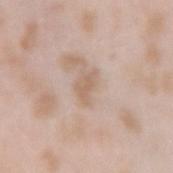Background:
Automated image analysis of the tile measured a mean CIELAB color near L≈62 a*≈16 b*≈28, roughly 8 lightness units darker than nearby skin, and a normalized lesion–skin contrast near 6. The software also gave a border-irregularity index near 4.5/10, a color-variation rating of about 1/10, and radial color variation of about 0.5. It also reported lesion-presence confidence of about 100/100. A roughly 15 mm field-of-view crop from a total-body skin photograph. Located on the arm. This is a white-light tile. The recorded lesion diameter is about 3 mm. The subject is a female about 25 years old.